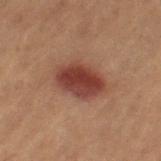workup = no biopsy performed (imaged during a skin exam)
subject = female, about 80 years old
image source = ~15 mm crop, total-body skin-cancer survey
site = the right thigh
size = about 5 mm